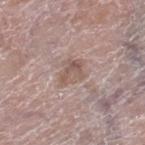The lesion was tiled from a total-body skin photograph and was not biopsied. The patient is a male aged approximately 80. A close-up tile cropped from a whole-body skin photograph, about 15 mm across. Imaged with white-light lighting. Located on the right lower leg.